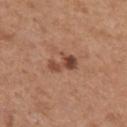follow-up: total-body-photography surveillance lesion; no biopsy
imaging modality: 15 mm crop, total-body photography
location: the right upper arm
subject: female, aged 28 to 32
size: about 4 mm
illumination: white-light illumination
TBP lesion metrics: an automated nevus-likeness rating near 40 out of 100 and a detector confidence of about 100 out of 100 that the crop contains a lesion